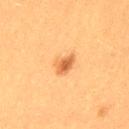Context:
The lesion is located on the left thigh. This is a cross-polarized tile. A 15 mm close-up tile from a total-body photography series done for melanoma screening. The total-body-photography lesion software estimated a detector confidence of about 100 out of 100 that the crop contains a lesion. The recorded lesion diameter is about 3 mm. A female subject, roughly 40 years of age.